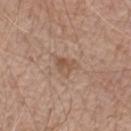<tbp_lesion>
<biopsy_status>not biopsied; imaged during a skin examination</biopsy_status>
<image>
  <source>total-body photography crop</source>
  <field_of_view_mm>15</field_of_view_mm>
</image>
<patient>
  <sex>male</sex>
  <age_approx>60</age_approx>
</patient>
<lighting>white-light</lighting>
<lesion_size>
  <long_diameter_mm_approx>3.0</long_diameter_mm_approx>
</lesion_size>
<site>left forearm</site>
</tbp_lesion>